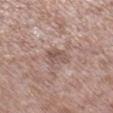The lesion was tiled from a total-body skin photograph and was not biopsied. Longest diameter approximately 3 mm. This is a white-light tile. A lesion tile, about 15 mm wide, cut from a 3D total-body photograph. On the right lower leg. A male subject, approximately 55 years of age.